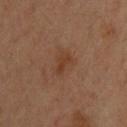{"biopsy_status": "not biopsied; imaged during a skin examination", "patient": {"sex": "male", "age_approx": 35}, "image": {"source": "total-body photography crop", "field_of_view_mm": 15}, "site": "upper back"}